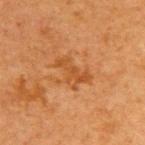Clinical impression: The lesion was tiled from a total-body skin photograph and was not biopsied. Acquisition and patient details: The subject is a female aged 38–42. Measured at roughly 4 mm in maximum diameter. Automated tile analysis of the lesion measured an outline eccentricity of about 0.85 (0 = round, 1 = elongated) and a symmetry-axis asymmetry near 0.45. The analysis additionally found about 7 CIELAB-L* units darker than the surrounding skin and a normalized border contrast of about 6. The analysis additionally found a border-irregularity index near 6/10, internal color variation of about 2.5 on a 0–10 scale, and a peripheral color-asymmetry measure near 1. A region of skin cropped from a whole-body photographic capture, roughly 15 mm wide. The tile uses cross-polarized illumination. On the upper back.